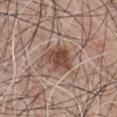image source: 15 mm crop, total-body photography
site: the chest
subject: male, roughly 75 years of age